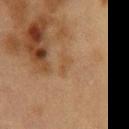follow-up=no biopsy performed (imaged during a skin exam)
body site=the chest
imaging modality=~15 mm tile from a whole-body skin photo
lesion diameter=about 2.5 mm
patient=female, in their 40s
tile lighting=cross-polarized